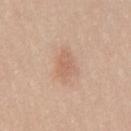Part of a total-body skin-imaging series; this lesion was reviewed on a skin check and was not flagged for biopsy.
A 15 mm crop from a total-body photograph taken for skin-cancer surveillance.
The lesion is located on the mid back.
The tile uses white-light illumination.
About 3 mm across.
Automated tile analysis of the lesion measured a border-irregularity rating of about 3/10, a within-lesion color-variation index near 2/10, and a peripheral color-asymmetry measure near 0.5. It also reported an automated nevus-likeness rating near 5 out of 100 and lesion-presence confidence of about 100/100.
A male patient aged 33 to 37.